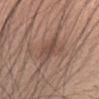biopsy status = imaged on a skin check; not biopsied | tile lighting = white-light | subject = female, aged around 30 | imaging modality = total-body-photography crop, ~15 mm field of view | site = the head or neck | diameter = about 3.5 mm.